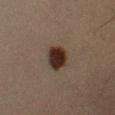<record>
<biopsy_status>not biopsied; imaged during a skin examination</biopsy_status>
<site>left lower leg</site>
<lesion_size>
  <long_diameter_mm_approx>3.0</long_diameter_mm_approx>
</lesion_size>
<patient>
  <sex>male</sex>
  <age_approx>40</age_approx>
</patient>
<image>
  <source>total-body photography crop</source>
  <field_of_view_mm>15</field_of_view_mm>
</image>
<lighting>cross-polarized</lighting>
</record>